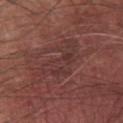| feature | finding |
|---|---|
| notes | no biopsy performed (imaged during a skin exam) |
| image source | ~15 mm crop, total-body skin-cancer survey |
| image-analysis metrics | a footprint of about 14 mm², an eccentricity of roughly 0.85, and a symmetry-axis asymmetry near 0.55; lesion-presence confidence of about 70/100 |
| diameter | about 6.5 mm |
| body site | the chest |
| patient | male, roughly 60 years of age |
| illumination | white-light |